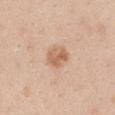• workup: no biopsy performed (imaged during a skin exam)
• patient: male, in their mid- to late 30s
• lesion size: ≈3 mm
• lighting: white-light illumination
• automated lesion analysis: two-axis asymmetry of about 0.2; a border-irregularity index near 2/10 and radial color variation of about 1.5; a classifier nevus-likeness of about 30/100
• anatomic site: the right upper arm
• image source: 15 mm crop, total-body photography